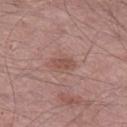{"lighting": "white-light", "image": {"source": "total-body photography crop", "field_of_view_mm": 15}, "patient": {"sex": "male", "age_approx": 65}, "automated_metrics": {"area_mm2_approx": 4.0, "eccentricity": 0.85, "shape_asymmetry": 0.25, "cielab_L": 51, "cielab_a": 21, "cielab_b": 24, "vs_skin_darker_L": 8.0, "vs_skin_contrast_norm": 6.0}, "site": "right lower leg"}